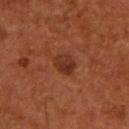| feature | finding |
|---|---|
| biopsy status | no biopsy performed (imaged during a skin exam) |
| image-analysis metrics | a footprint of about 5 mm² and a shape eccentricity near 0.6; an average lesion color of about L≈29 a*≈24 b*≈28 (CIELAB), roughly 8 lightness units darker than nearby skin, and a lesion-to-skin contrast of about 7.5 (normalized; higher = more distinct); a lesion-detection confidence of about 100/100 |
| lesion diameter | about 3 mm |
| body site | the upper back |
| lighting | cross-polarized illumination |
| image source | ~15 mm crop, total-body skin-cancer survey |
| subject | male, about 55 years old |